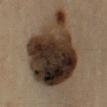| field | value |
|---|---|
| notes | no biopsy performed (imaged during a skin exam) |
| location | the right upper arm |
| diameter | ≈10 mm |
| image-analysis metrics | an area of roughly 60 mm², an eccentricity of roughly 0.65, and a symmetry-axis asymmetry near 0.25; a border-irregularity index near 3/10, a color-variation rating of about 10/10, and a peripheral color-asymmetry measure near 4 |
| acquisition | ~15 mm tile from a whole-body skin photo |
| patient | male, aged 53 to 57 |